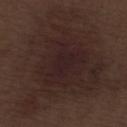Recorded during total-body skin imaging; not selected for excision or biopsy. A 15 mm crop from a total-body photograph taken for skin-cancer surveillance. On the left lower leg. A male patient aged around 70. This is a white-light tile. Approximately 15 mm at its widest.